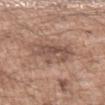Part of a total-body skin-imaging series; this lesion was reviewed on a skin check and was not flagged for biopsy. A male subject, roughly 50 years of age. Captured under white-light illumination. The lesion-visualizer software estimated a border-irregularity rating of about 4.5/10 and peripheral color asymmetry of about 2. And it measured a classifier nevus-likeness of about 0/100 and a lesion-detection confidence of about 100/100. Located on the left upper arm. A 15 mm crop from a total-body photograph taken for skin-cancer surveillance.